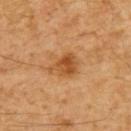The lesion was photographed on a routine skin check and not biopsied; there is no pathology result. From the upper back. Captured under cross-polarized illumination. A male patient approximately 60 years of age. Longest diameter approximately 3.5 mm. A region of skin cropped from a whole-body photographic capture, roughly 15 mm wide.